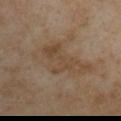Q: Was a biopsy performed?
A: imaged on a skin check; not biopsied
Q: What is the imaging modality?
A: ~15 mm tile from a whole-body skin photo
Q: How was the tile lit?
A: cross-polarized
Q: What is the lesion's diameter?
A: ≈6.5 mm
Q: What is the anatomic site?
A: the right upper arm
Q: Who is the patient?
A: male, in their mid-50s